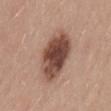Q: Is there a histopathology result?
A: catalogued during a skin exam; not biopsied
Q: How was this image acquired?
A: total-body-photography crop, ~15 mm field of view
Q: Where on the body is the lesion?
A: the lower back
Q: Who is the patient?
A: male, in their mid- to late 30s
Q: Lesion size?
A: ≈6.5 mm
Q: What did automated image analysis measure?
A: border irregularity of about 2 on a 0–10 scale and a peripheral color-asymmetry measure near 1.5; a classifier nevus-likeness of about 55/100
Q: How was the tile lit?
A: white-light